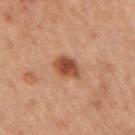notes = total-body-photography surveillance lesion; no biopsy
subject = female, aged 43 to 47
acquisition = total-body-photography crop, ~15 mm field of view
automated lesion analysis = a border-irregularity rating of about 2/10, a within-lesion color-variation index near 4/10, and a peripheral color-asymmetry measure near 1
anatomic site = the right upper arm
diameter = ≈3.5 mm
illumination = cross-polarized